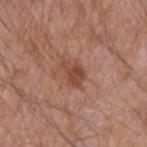{
  "biopsy_status": "not biopsied; imaged during a skin examination"
}